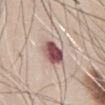Impression: The lesion was photographed on a routine skin check and not biopsied; there is no pathology result. Clinical summary: The lesion is on the abdomen. The total-body-photography lesion software estimated a lesion area of about 8 mm², an outline eccentricity of about 0.6 (0 = round, 1 = elongated), and a symmetry-axis asymmetry near 0.2. The analysis additionally found a detector confidence of about 100 out of 100 that the crop contains a lesion. Captured under white-light illumination. The recorded lesion diameter is about 3.5 mm. A roughly 15 mm field-of-view crop from a total-body skin photograph. A male patient aged 63 to 67.